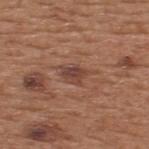follow-up: total-body-photography surveillance lesion; no biopsy | acquisition: ~15 mm crop, total-body skin-cancer survey | automated metrics: an average lesion color of about L≈43 a*≈21 b*≈26 (CIELAB), a lesion–skin lightness drop of about 8, and a normalized border contrast of about 7; a border-irregularity rating of about 3.5/10 and internal color variation of about 4 on a 0–10 scale | illumination: white-light | patient: male, aged 53–57 | site: the back.